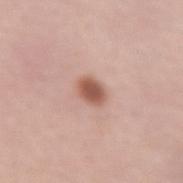The lesion was tiled from a total-body skin photograph and was not biopsied. An algorithmic analysis of the crop reported a footprint of about 5 mm², an eccentricity of roughly 0.65, and two-axis asymmetry of about 0.15. And it measured a lesion color around L≈56 a*≈23 b*≈27 in CIELAB, about 14 CIELAB-L* units darker than the surrounding skin, and a normalized border contrast of about 9. The software also gave an automated nevus-likeness rating near 100 out of 100 and a lesion-detection confidence of about 100/100. Located on the left forearm. A roughly 15 mm field-of-view crop from a total-body skin photograph. A female patient aged 58–62.